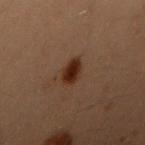notes=catalogued during a skin exam; not biopsied
subject=female, approximately 40 years of age
site=the right upper arm
lesion diameter=≈2.5 mm
automated lesion analysis=border irregularity of about 1.5 on a 0–10 scale and a peripheral color-asymmetry measure near 0.5
image=15 mm crop, total-body photography